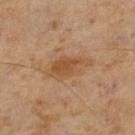notes: no biopsy performed (imaged during a skin exam); tile lighting: cross-polarized illumination; subject: male, about 60 years old; image source: ~15 mm crop, total-body skin-cancer survey; body site: the left lower leg.